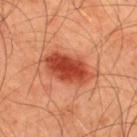{"biopsy_status": "not biopsied; imaged during a skin examination", "patient": {"sex": "male", "age_approx": 45}, "lighting": "cross-polarized", "site": "upper back", "image": {"source": "total-body photography crop", "field_of_view_mm": 15}, "automated_metrics": {"area_mm2_approx": 19.0, "eccentricity": 0.85, "shape_asymmetry": 0.2, "cielab_L": 48, "cielab_a": 32, "cielab_b": 35, "vs_skin_darker_L": 14.0, "vs_skin_contrast_norm": 9.5}}